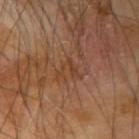A 15 mm close-up tile from a total-body photography series done for melanoma screening. Imaged with cross-polarized lighting. The recorded lesion diameter is about 3 mm. A male subject, aged 63–67. The lesion is located on the arm.